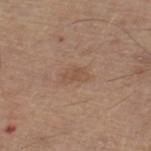Captured during whole-body skin photography for melanoma surveillance; the lesion was not biopsied. The subject is a male in their mid-60s. Automated image analysis of the tile measured a footprint of about 3.5 mm², an outline eccentricity of about 0.85 (0 = round, 1 = elongated), and a symmetry-axis asymmetry near 0.3. And it measured a lesion color around L≈50 a*≈18 b*≈29 in CIELAB, about 6 CIELAB-L* units darker than the surrounding skin, and a lesion-to-skin contrast of about 5 (normalized; higher = more distinct). It also reported a border-irregularity index near 3/10 and a peripheral color-asymmetry measure near 0.5. Captured under white-light illumination. A roughly 15 mm field-of-view crop from a total-body skin photograph. Approximately 3 mm at its widest. From the right thigh.